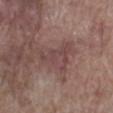patient: male, approximately 70 years of age
size: ≈4.5 mm
body site: the right lower leg
image: ~15 mm tile from a whole-body skin photo
tile lighting: white-light illumination